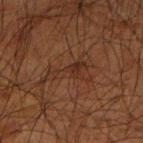Q: Is there a histopathology result?
A: total-body-photography surveillance lesion; no biopsy
Q: What lighting was used for the tile?
A: cross-polarized illumination
Q: Lesion size?
A: ≈6.5 mm
Q: Who is the patient?
A: male, in their mid-60s
Q: Lesion location?
A: the right upper arm
Q: What is the imaging modality?
A: ~15 mm tile from a whole-body skin photo